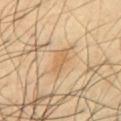Clinical impression:
Part of a total-body skin-imaging series; this lesion was reviewed on a skin check and was not flagged for biopsy.
Background:
An algorithmic analysis of the crop reported a lesion area of about 4 mm² and an eccentricity of roughly 0.9. It also reported a border-irregularity rating of about 3.5/10, internal color variation of about 1 on a 0–10 scale, and radial color variation of about 0.5. Located on the chest. A male patient, aged approximately 40. The lesion's longest dimension is about 3.5 mm. This image is a 15 mm lesion crop taken from a total-body photograph.